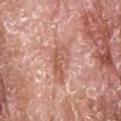| field | value |
|---|---|
| follow-up | catalogued during a skin exam; not biopsied |
| image-analysis metrics | an eccentricity of roughly 0.9 |
| patient | male, approximately 65 years of age |
| image source | total-body-photography crop, ~15 mm field of view |
| illumination | white-light |
| location | the head or neck |
| lesion size | ~5 mm (longest diameter) |